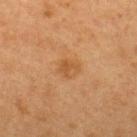Part of a total-body skin-imaging series; this lesion was reviewed on a skin check and was not flagged for biopsy. A female patient, aged around 40. Automated image analysis of the tile measured a shape eccentricity near 0.7 and two-axis asymmetry of about 0.2. And it measured a nevus-likeness score of about 10/100 and a lesion-detection confidence of about 100/100. This is a cross-polarized tile. The lesion's longest dimension is about 3 mm. The lesion is on the upper back. Cropped from a total-body skin-imaging series; the visible field is about 15 mm.